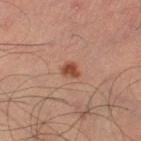Notes:
– patient — male, in their mid- to late 30s
– location — the left thigh
– image source — 15 mm crop, total-body photography